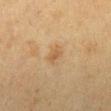follow-up=total-body-photography surveillance lesion; no biopsy | image-analysis metrics=a lesion color around L≈49 a*≈14 b*≈33 in CIELAB, about 6 CIELAB-L* units darker than the surrounding skin, and a normalized border contrast of about 5; border irregularity of about 3 on a 0–10 scale, internal color variation of about 2 on a 0–10 scale, and radial color variation of about 0.5; a nevus-likeness score of about 30/100 and lesion-presence confidence of about 100/100 | diameter=about 2.5 mm | subject=female, aged approximately 55 | body site=the right lower leg | tile lighting=cross-polarized | image=~15 mm tile from a whole-body skin photo.